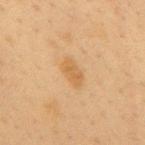follow-up = total-body-photography surveillance lesion; no biopsy
subject = female, roughly 40 years of age
acquisition = total-body-photography crop, ~15 mm field of view
diameter = about 3.5 mm
lighting = cross-polarized
site = the mid back